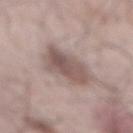Assessment:
Imaged during a routine full-body skin examination; the lesion was not biopsied and no histopathology is available.
Context:
This is a white-light tile. From the mid back. Automated image analysis of the tile measured a border-irregularity rating of about 2.5/10, a color-variation rating of about 4.5/10, and peripheral color asymmetry of about 1.5. It also reported a classifier nevus-likeness of about 55/100. About 5 mm across. A male subject aged around 65. A 15 mm close-up tile from a total-body photography series done for melanoma screening.